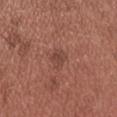Captured during whole-body skin photography for melanoma surveillance; the lesion was not biopsied. Located on the head or neck. A 15 mm close-up extracted from a 3D total-body photography capture. A male patient aged 23–27.